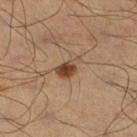location = the left leg; acquisition = 15 mm crop, total-body photography; patient = male, aged around 50; size = ~3 mm (longest diameter); lighting = cross-polarized.